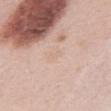  biopsy_status: not biopsied; imaged during a skin examination
  image:
    source: total-body photography crop
    field_of_view_mm: 15
  lesion_size:
    long_diameter_mm_approx: 2.0
  lighting: white-light
  site: chest
  patient:
    sex: female
    age_approx: 50
  automated_metrics:
    area_mm2_approx: 1.5
    eccentricity: 0.85
    shape_asymmetry: 0.35
    cielab_L: 70
    cielab_a: 15
    cielab_b: 29
    vs_skin_contrast_norm: 3.0
    nevus_likeness_0_100: 0